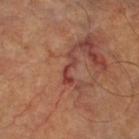| feature | finding |
|---|---|
| anatomic site | the right thigh |
| subject | in their mid-60s |
| tile lighting | cross-polarized illumination |
| imaging modality | 15 mm crop, total-body photography |